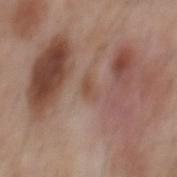Recorded during total-body skin imaging; not selected for excision or biopsy. The lesion-visualizer software estimated a lesion color around L≈50 a*≈19 b*≈27 in CIELAB, about 7 CIELAB-L* units darker than the surrounding skin, and a normalized lesion–skin contrast near 6. The analysis additionally found an automated nevus-likeness rating near 0 out of 100 and lesion-presence confidence of about 95/100. A male subject aged 53 to 57. Approximately 2.5 mm at its widest. From the mid back. A 15 mm close-up extracted from a 3D total-body photography capture. This is a white-light tile.